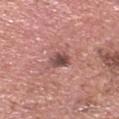| key | value |
|---|---|
| notes | imaged on a skin check; not biopsied |
| illumination | white-light |
| anatomic site | the head or neck |
| image source | ~15 mm tile from a whole-body skin photo |
| lesion diameter | ≈2.5 mm |
| TBP lesion metrics | an outline eccentricity of about 0.5 (0 = round, 1 = elongated) and a symmetry-axis asymmetry near 0.3; a within-lesion color-variation index near 5.5/10 and radial color variation of about 2; a nevus-likeness score of about 40/100 and a lesion-detection confidence of about 100/100 |
| patient | male, in their mid-60s |